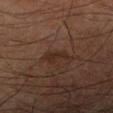Findings:
* biopsy status · total-body-photography surveillance lesion; no biopsy
* image source · ~15 mm crop, total-body skin-cancer survey
* site · the left lower leg
* diameter · about 3 mm
* patient · male, aged 63 to 67
* tile lighting · cross-polarized